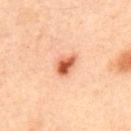tile lighting = cross-polarized illumination; acquisition = total-body-photography crop, ~15 mm field of view; automated metrics = a footprint of about 5.5 mm², a shape eccentricity near 0.75, and two-axis asymmetry of about 0.25; patient = male, approximately 35 years of age; location = the upper back.